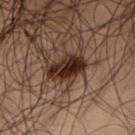Assessment: This lesion was catalogued during total-body skin photography and was not selected for biopsy. Image and clinical context: The total-body-photography lesion software estimated an outline eccentricity of about 0.85 (0 = round, 1 = elongated) and two-axis asymmetry of about 0.25. It also reported a nevus-likeness score of about 95/100 and a detector confidence of about 100 out of 100 that the crop contains a lesion. A region of skin cropped from a whole-body photographic capture, roughly 15 mm wide. Longest diameter approximately 4.5 mm. The lesion is located on the right thigh. A male patient, aged 48–52.